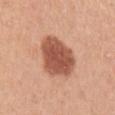patient = female, aged 43 to 47 | imaging modality = 15 mm crop, total-body photography | body site = the left upper arm | image-analysis metrics = a lesion–skin lightness drop of about 16 and a normalized border contrast of about 10; a border-irregularity index near 2/10, internal color variation of about 3.5 on a 0–10 scale, and peripheral color asymmetry of about 1 | lesion size = ~5 mm (longest diameter) | lighting = white-light illumination.